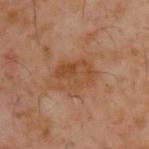The lesion was photographed on a routine skin check and not biopsied; there is no pathology result. Longest diameter approximately 4.5 mm. A male patient aged approximately 60. A 15 mm close-up extracted from a 3D total-body photography capture. The tile uses cross-polarized illumination. From the upper back.